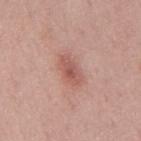No biopsy was performed on this lesion — it was imaged during a full skin examination and was not determined to be concerning.
The lesion is on the mid back.
Automated tile analysis of the lesion measured a lesion–skin lightness drop of about 10 and a normalized lesion–skin contrast near 6.5. And it measured border irregularity of about 3 on a 0–10 scale, internal color variation of about 3 on a 0–10 scale, and radial color variation of about 1. The software also gave a nevus-likeness score of about 85/100 and a lesion-detection confidence of about 100/100.
A male subject about 45 years old.
A roughly 15 mm field-of-view crop from a total-body skin photograph.